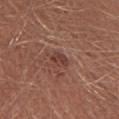Captured during whole-body skin photography for melanoma surveillance; the lesion was not biopsied. From the left thigh. Cropped from a total-body skin-imaging series; the visible field is about 15 mm. The total-body-photography lesion software estimated a lesion area of about 3 mm², a shape eccentricity near 0.8, and a symmetry-axis asymmetry near 0.25. And it measured a lesion color around L≈40 a*≈23 b*≈25 in CIELAB, about 8 CIELAB-L* units darker than the surrounding skin, and a normalized lesion–skin contrast near 7. Measured at roughly 2.5 mm in maximum diameter. The patient is a male aged approximately 30.